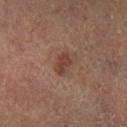Q: Was this lesion biopsied?
A: no biopsy performed (imaged during a skin exam)
Q: Lesion location?
A: the leg
Q: What are the patient's age and sex?
A: male, in their mid- to late 60s
Q: Lesion size?
A: ~3 mm (longest diameter)
Q: How was the tile lit?
A: cross-polarized
Q: How was this image acquired?
A: total-body-photography crop, ~15 mm field of view